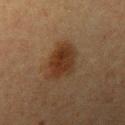{
  "biopsy_status": "not biopsied; imaged during a skin examination",
  "site": "left upper arm",
  "image": {
    "source": "total-body photography crop",
    "field_of_view_mm": 15
  },
  "automated_metrics": {
    "shape_asymmetry": 0.25,
    "cielab_L": 27,
    "cielab_a": 15,
    "cielab_b": 25,
    "vs_skin_darker_L": 8.0,
    "vs_skin_contrast_norm": 9.0,
    "nevus_likeness_0_100": 95,
    "lesion_detection_confidence_0_100": 100
  },
  "patient": {
    "sex": "female",
    "age_approx": 55
  },
  "lesion_size": {
    "long_diameter_mm_approx": 5.0
  }
}